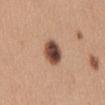biopsy status = no biopsy performed (imaged during a skin exam) | body site = the abdomen | automated metrics = a color-variation rating of about 8/10 and a peripheral color-asymmetry measure near 2.5; a detector confidence of about 100 out of 100 that the crop contains a lesion | subject = female, approximately 30 years of age | tile lighting = white-light | image source = ~15 mm crop, total-body skin-cancer survey | lesion diameter = about 3.5 mm.